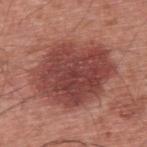The lesion was tiled from a total-body skin photograph and was not biopsied. A region of skin cropped from a whole-body photographic capture, roughly 15 mm wide. The subject is a male aged 58 to 62. On the upper back.